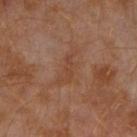illumination: cross-polarized | diameter: ~4.5 mm (longest diameter) | body site: the arm | patient: male, approximately 30 years of age | acquisition: total-body-photography crop, ~15 mm field of view | automated lesion analysis: a footprint of about 5.5 mm² and a shape-asymmetry score of about 0.4 (0 = symmetric); border irregularity of about 5.5 on a 0–10 scale, a within-lesion color-variation index near 1.5/10, and peripheral color asymmetry of about 0.5; a nevus-likeness score of about 0/100.